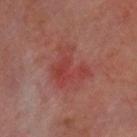Impression:
No biopsy was performed on this lesion — it was imaged during a full skin examination and was not determined to be concerning.
Background:
The lesion-visualizer software estimated a classifier nevus-likeness of about 0/100 and lesion-presence confidence of about 100/100. The tile uses cross-polarized illumination. The lesion's longest dimension is about 5 mm. A male patient, aged around 60. On the head or neck. A 15 mm close-up extracted from a 3D total-body photography capture.